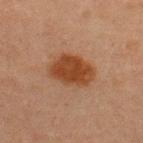workup=total-body-photography surveillance lesion; no biopsy
diameter=~5 mm (longest diameter)
subject=male, in their 60s
imaging modality=~15 mm crop, total-body skin-cancer survey
site=the upper back